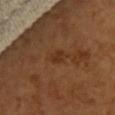{
  "biopsy_status": "not biopsied; imaged during a skin examination",
  "lesion_size": {
    "long_diameter_mm_approx": 2.0
  },
  "automated_metrics": {
    "area_mm2_approx": 3.0,
    "eccentricity": 0.6,
    "shape_asymmetry": 0.2,
    "border_irregularity_0_10": 2.0,
    "color_variation_0_10": 1.5
  },
  "site": "front of the torso",
  "image": {
    "source": "total-body photography crop",
    "field_of_view_mm": 15
  },
  "patient": {
    "sex": "female",
    "age_approx": 55
  }
}